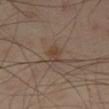Clinical impression: Part of a total-body skin-imaging series; this lesion was reviewed on a skin check and was not flagged for biopsy. Clinical summary: A region of skin cropped from a whole-body photographic capture, roughly 15 mm wide. On the left thigh. A male patient about 55 years old. Automated tile analysis of the lesion measured a lesion area of about 2.5 mm², a shape eccentricity near 0.9, and a symmetry-axis asymmetry near 0.3. The analysis additionally found a nevus-likeness score of about 10/100 and a lesion-detection confidence of about 100/100. Measured at roughly 2.5 mm in maximum diameter.